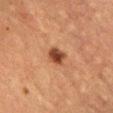Findings:
* workup: total-body-photography surveillance lesion; no biopsy
* acquisition: ~15 mm crop, total-body skin-cancer survey
* patient: female, aged approximately 55
* TBP lesion metrics: an average lesion color of about L≈36 a*≈21 b*≈29 (CIELAB), a lesion–skin lightness drop of about 13, and a lesion-to-skin contrast of about 11 (normalized; higher = more distinct); border irregularity of about 1.5 on a 0–10 scale and a color-variation rating of about 3.5/10; an automated nevus-likeness rating near 95 out of 100 and a lesion-detection confidence of about 100/100
* diameter: about 2.5 mm
* anatomic site: the chest
* illumination: cross-polarized illumination